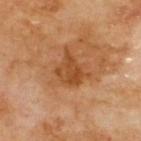Clinical impression:
No biopsy was performed on this lesion — it was imaged during a full skin examination and was not determined to be concerning.